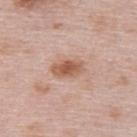Captured during whole-body skin photography for melanoma surveillance; the lesion was not biopsied.
The lesion is located on the upper back.
The patient is a female aged 63–67.
Imaged with white-light lighting.
A 15 mm close-up tile from a total-body photography series done for melanoma screening.
Longest diameter approximately 3.5 mm.